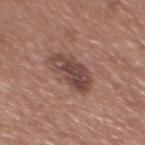Recorded during total-body skin imaging; not selected for excision or biopsy.
A lesion tile, about 15 mm wide, cut from a 3D total-body photograph.
A male patient approximately 45 years of age.
From the front of the torso.